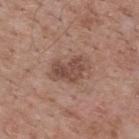<lesion>
<biopsy_status>not biopsied; imaged during a skin examination</biopsy_status>
<site>upper back</site>
<image>
  <source>total-body photography crop</source>
  <field_of_view_mm>15</field_of_view_mm>
</image>
<patient>
  <sex>male</sex>
  <age_approx>70</age_approx>
</patient>
<lesion_size>
  <long_diameter_mm_approx>4.5</long_diameter_mm_approx>
</lesion_size>
<lighting>white-light</lighting>
</lesion>